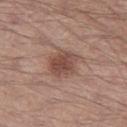Assessment:
The lesion was photographed on a routine skin check and not biopsied; there is no pathology result.
Context:
The tile uses white-light illumination. A male patient, aged approximately 40. From the left thigh. Cropped from a total-body skin-imaging series; the visible field is about 15 mm.